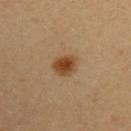Recorded during total-body skin imaging; not selected for excision or biopsy.
The lesion-visualizer software estimated a lesion area of about 6 mm², an outline eccentricity of about 0.5 (0 = round, 1 = elongated), and a symmetry-axis asymmetry near 0.2. It also reported border irregularity of about 2 on a 0–10 scale and a within-lesion color-variation index near 5.5/10.
The patient is a female roughly 40 years of age.
The tile uses cross-polarized illumination.
Approximately 3 mm at its widest.
On the left upper arm.
A 15 mm close-up tile from a total-body photography series done for melanoma screening.